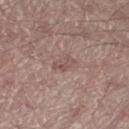| feature | finding |
|---|---|
| workup | no biopsy performed (imaged during a skin exam) |
| image | ~15 mm tile from a whole-body skin photo |
| anatomic site | the right thigh |
| patient | male, aged approximately 55 |
| lesion size | ≈2.5 mm |
| TBP lesion metrics | a footprint of about 3 mm², an outline eccentricity of about 0.85 (0 = round, 1 = elongated), and a symmetry-axis asymmetry near 0.45; internal color variation of about 0 on a 0–10 scale and a peripheral color-asymmetry measure near 0 |